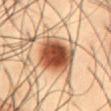{"site": "abdomen", "image": {"source": "total-body photography crop", "field_of_view_mm": 15}, "patient": {"sex": "male", "age_approx": 55}}